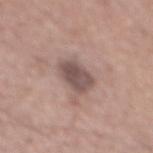follow-up — total-body-photography surveillance lesion; no biopsy
lesion diameter — ~4 mm (longest diameter)
patient — male, aged 53 to 57
location — the mid back
tile lighting — white-light
image source — ~15 mm crop, total-body skin-cancer survey